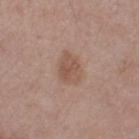biopsy status: imaged on a skin check; not biopsied
imaging modality: ~15 mm crop, total-body skin-cancer survey
subject: male, roughly 75 years of age
diameter: about 4 mm
anatomic site: the right thigh
lighting: white-light illumination
automated metrics: a lesion area of about 8.5 mm² and an outline eccentricity of about 0.7 (0 = round, 1 = elongated); a border-irregularity index near 2/10, a color-variation rating of about 3/10, and radial color variation of about 1